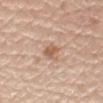<case>
  <biopsy_status>not biopsied; imaged during a skin examination</biopsy_status>
  <patient>
    <sex>male</sex>
    <age_approx>60</age_approx>
  </patient>
  <site>right forearm</site>
  <image>
    <source>total-body photography crop</source>
    <field_of_view_mm>15</field_of_view_mm>
  </image>
  <automated_metrics>
    <eccentricity>0.75</eccentricity>
    <shape_asymmetry>0.2</shape_asymmetry>
    <cielab_L>59</cielab_L>
    <cielab_a>20</cielab_a>
    <cielab_b>31</cielab_b>
    <vs_skin_darker_L>10.0</vs_skin_darker_L>
    <vs_skin_contrast_norm>7.0</vs_skin_contrast_norm>
    <border_irregularity_0_10>2.0</border_irregularity_0_10>
    <color_variation_0_10>2.5</color_variation_0_10>
    <peripheral_color_asymmetry>1.0</peripheral_color_asymmetry>
    <nevus_likeness_0_100>5</nevus_likeness_0_100>
    <lesion_detection_confidence_0_100>100</lesion_detection_confidence_0_100>
  </automated_metrics>
  <lighting>white-light</lighting>
  <lesion_size>
    <long_diameter_mm_approx>2.5</long_diameter_mm_approx>
  </lesion_size>
</case>